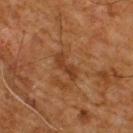Case summary:
* follow-up — imaged on a skin check; not biopsied
* image source — ~15 mm crop, total-body skin-cancer survey
* TBP lesion metrics — a mean CIELAB color near L≈32 a*≈20 b*≈30, about 7 CIELAB-L* units darker than the surrounding skin, and a lesion-to-skin contrast of about 6.5 (normalized; higher = more distinct); a color-variation rating of about 1/10 and a peripheral color-asymmetry measure near 0.5; a nevus-likeness score of about 0/100
* diameter — ~3.5 mm (longest diameter)
* site — the upper back
* subject — male, roughly 60 years of age
* lighting — cross-polarized illumination